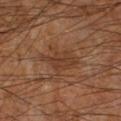<tbp_lesion>
<biopsy_status>not biopsied; imaged during a skin examination</biopsy_status>
<image>
  <source>total-body photography crop</source>
  <field_of_view_mm>15</field_of_view_mm>
</image>
<site>right lower leg</site>
<patient>
  <sex>male</sex>
  <age_approx>60</age_approx>
</patient>
<lesion_size>
  <long_diameter_mm_approx>5.0</long_diameter_mm_approx>
</lesion_size>
<lighting>cross-polarized</lighting>
</tbp_lesion>